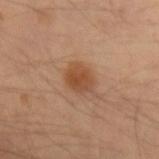biopsy status=catalogued during a skin exam; not biopsied.